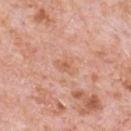Captured during whole-body skin photography for melanoma surveillance; the lesion was not biopsied. The total-body-photography lesion software estimated internal color variation of about 1.5 on a 0–10 scale and peripheral color asymmetry of about 0.5. A male patient, aged 78 to 82. The lesion is located on the chest. Longest diameter approximately 2.5 mm. A lesion tile, about 15 mm wide, cut from a 3D total-body photograph.